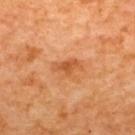follow-up = no biopsy performed (imaged during a skin exam)
acquisition = ~15 mm tile from a whole-body skin photo
subject = female, aged around 65
body site = the back
tile lighting = cross-polarized illumination
lesion size = ≈3.5 mm
image-analysis metrics = a lesion area of about 4.5 mm² and a shape eccentricity near 0.85; a mean CIELAB color near L≈54 a*≈28 b*≈43, a lesion–skin lightness drop of about 9, and a normalized lesion–skin contrast near 6.5; a classifier nevus-likeness of about 0/100 and a detector confidence of about 100 out of 100 that the crop contains a lesion